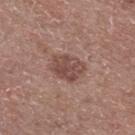Imaged during a routine full-body skin examination; the lesion was not biopsied and no histopathology is available. The lesion is on the upper back. Captured under white-light illumination. A male subject, approximately 60 years of age. A 15 mm close-up tile from a total-body photography series done for melanoma screening. The lesion-visualizer software estimated an outline eccentricity of about 0.7 (0 = round, 1 = elongated). It also reported a border-irregularity rating of about 2/10 and peripheral color asymmetry of about 1.5. It also reported a detector confidence of about 100 out of 100 that the crop contains a lesion.